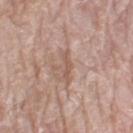{
  "biopsy_status": "not biopsied; imaged during a skin examination",
  "patient": {
    "sex": "female",
    "age_approx": 75
  },
  "site": "leg",
  "image": {
    "source": "total-body photography crop",
    "field_of_view_mm": 15
  }
}